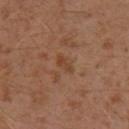follow-up = imaged on a skin check; not biopsied
lesion size = ≈2.5 mm
imaging modality = ~15 mm crop, total-body skin-cancer survey
patient = male, aged approximately 30
body site = the left lower leg